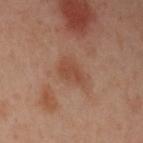The lesion was photographed on a routine skin check and not biopsied; there is no pathology result. Cropped from a total-body skin-imaging series; the visible field is about 15 mm. A female patient, aged around 40. The lesion is located on the left upper arm. Imaged with cross-polarized lighting.